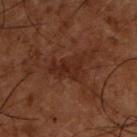Longest diameter approximately 5 mm. A 15 mm close-up extracted from a 3D total-body photography capture. Located on the back. A male subject about 50 years old. The tile uses cross-polarized illumination. Automated tile analysis of the lesion measured an average lesion color of about L≈19 a*≈17 b*≈20 (CIELAB), about 5 CIELAB-L* units darker than the surrounding skin, and a normalized lesion–skin contrast near 6.5. The software also gave an automated nevus-likeness rating near 5 out of 100 and a detector confidence of about 85 out of 100 that the crop contains a lesion.